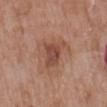Q: Is there a histopathology result?
A: no biopsy performed (imaged during a skin exam)
Q: What kind of image is this?
A: ~15 mm tile from a whole-body skin photo
Q: What is the lesion's diameter?
A: about 4 mm
Q: Lesion location?
A: the back
Q: How was the tile lit?
A: white-light
Q: What are the patient's age and sex?
A: female, roughly 75 years of age